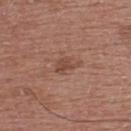Q: Was this lesion biopsied?
A: imaged on a skin check; not biopsied
Q: What is the anatomic site?
A: the back
Q: Who is the patient?
A: male, approximately 55 years of age
Q: What kind of image is this?
A: total-body-photography crop, ~15 mm field of view